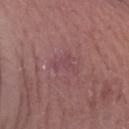{"biopsy_status": "not biopsied; imaged during a skin examination", "patient": {"sex": "male", "age_approx": 55}, "site": "left forearm", "image": {"source": "total-body photography crop", "field_of_view_mm": 15}}